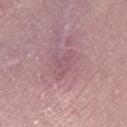biopsy status: no biopsy performed (imaged during a skin exam) | patient: female, aged approximately 60 | illumination: white-light illumination | acquisition: 15 mm crop, total-body photography | lesion diameter: ≈4 mm | anatomic site: the right thigh.